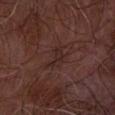Case summary:
- follow-up — imaged on a skin check; not biopsied
- body site — the left forearm
- image source — ~15 mm crop, total-body skin-cancer survey
- subject — male, aged 63 to 67